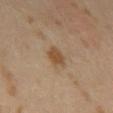{
  "biopsy_status": "not biopsied; imaged during a skin examination",
  "lesion_size": {
    "long_diameter_mm_approx": 3.0
  },
  "automated_metrics": {
    "cielab_L": 47,
    "cielab_a": 16,
    "cielab_b": 32,
    "vs_skin_darker_L": 9.0,
    "vs_skin_contrast_norm": 8.0,
    "border_irregularity_0_10": 2.0,
    "color_variation_0_10": 1.5,
    "peripheral_color_asymmetry": 0.5,
    "lesion_detection_confidence_0_100": 100
  },
  "lighting": "cross-polarized",
  "patient": {
    "sex": "male",
    "age_approx": 65
  },
  "image": {
    "source": "total-body photography crop",
    "field_of_view_mm": 15
  }
}